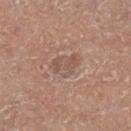<record>
<biopsy_status>not biopsied; imaged during a skin examination</biopsy_status>
<site>leg</site>
<patient>
  <sex>male</sex>
  <age_approx>75</age_approx>
</patient>
<image>
  <source>total-body photography crop</source>
  <field_of_view_mm>15</field_of_view_mm>
</image>
<lighting>white-light</lighting>
</record>